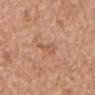The lesion was tiled from a total-body skin photograph and was not biopsied.
Cropped from a total-body skin-imaging series; the visible field is about 15 mm.
Located on the chest.
The subject is a male about 70 years old.